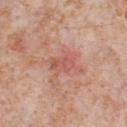Case summary:
– biopsy status — imaged on a skin check; not biopsied
– image — ~15 mm tile from a whole-body skin photo
– subject — male, aged around 65
– lesion size — ≈2.5 mm
– illumination — white-light illumination
– body site — the chest